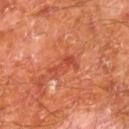The lesion was photographed on a routine skin check and not biopsied; there is no pathology result. A male subject, in their mid-60s. A region of skin cropped from a whole-body photographic capture, roughly 15 mm wide. Located on the leg.